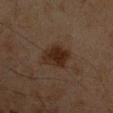The lesion was photographed on a routine skin check and not biopsied; there is no pathology result.